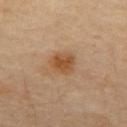notes — total-body-photography surveillance lesion; no biopsy | automated metrics — a footprint of about 7 mm² and a shape-asymmetry score of about 0.2 (0 = symmetric); a mean CIELAB color near L≈48 a*≈19 b*≈35, roughly 9 lightness units darker than nearby skin, and a lesion-to-skin contrast of about 8 (normalized; higher = more distinct); a border-irregularity index near 2/10; a nevus-likeness score of about 85/100 and a detector confidence of about 100 out of 100 that the crop contains a lesion | size — ≈3 mm | site — the mid back | subject — male, aged around 60 | acquisition — ~15 mm crop, total-body skin-cancer survey.